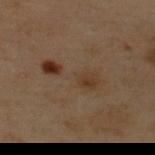notes=total-body-photography surveillance lesion; no biopsy | patient=male, aged approximately 55 | anatomic site=the upper back | lesion diameter=~7 mm (longest diameter) | TBP lesion metrics=border irregularity of about 7 on a 0–10 scale, internal color variation of about 5.5 on a 0–10 scale, and peripheral color asymmetry of about 1.5 | acquisition=total-body-photography crop, ~15 mm field of view | lighting=cross-polarized illumination.